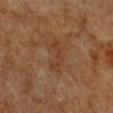Assessment:
Recorded during total-body skin imaging; not selected for excision or biopsy.
Image and clinical context:
The subject is a female roughly 55 years of age. Cropped from a total-body skin-imaging series; the visible field is about 15 mm. Imaged with cross-polarized lighting. On the chest. Automated tile analysis of the lesion measured a footprint of about 5 mm² and a symmetry-axis asymmetry near 0.55. It also reported a within-lesion color-variation index near 0/10 and radial color variation of about 0. The software also gave lesion-presence confidence of about 100/100. The recorded lesion diameter is about 4 mm.